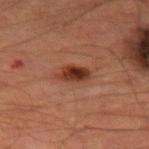Recorded during total-body skin imaging; not selected for excision or biopsy. The tile uses cross-polarized illumination. Located on the left thigh. A roughly 15 mm field-of-view crop from a total-body skin photograph. A male patient, aged around 65. Longest diameter approximately 3.5 mm. An algorithmic analysis of the crop reported a mean CIELAB color near L≈32 a*≈23 b*≈28, roughly 11 lightness units darker than nearby skin, and a normalized border contrast of about 10. The analysis additionally found a border-irregularity index near 2/10, a within-lesion color-variation index near 5.5/10, and radial color variation of about 2.